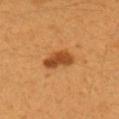Imaged during a routine full-body skin examination; the lesion was not biopsied and no histopathology is available. The lesion is located on the left forearm. A 15 mm close-up tile from a total-body photography series done for melanoma screening. Automated tile analysis of the lesion measured a footprint of about 7 mm² and a shape-asymmetry score of about 0.3 (0 = symmetric). The analysis additionally found an average lesion color of about L≈46 a*≈26 b*≈41 (CIELAB), about 13 CIELAB-L* units darker than the surrounding skin, and a normalized border contrast of about 9. It also reported border irregularity of about 3 on a 0–10 scale. The software also gave an automated nevus-likeness rating near 100 out of 100 and a detector confidence of about 100 out of 100 that the crop contains a lesion. The subject is a female aged approximately 25. Longest diameter approximately 3.5 mm. This is a cross-polarized tile.